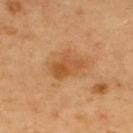Impression: Imaged during a routine full-body skin examination; the lesion was not biopsied and no histopathology is available. Clinical summary: From the upper back. A 15 mm crop from a total-body photograph taken for skin-cancer surveillance. A female subject, about 60 years old.